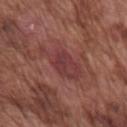| feature | finding |
|---|---|
| biopsy status | no biopsy performed (imaged during a skin exam) |
| image-analysis metrics | an average lesion color of about L≈39 a*≈26 b*≈21 (CIELAB), a lesion–skin lightness drop of about 7, and a normalized lesion–skin contrast near 6.5; border irregularity of about 5 on a 0–10 scale, internal color variation of about 3 on a 0–10 scale, and radial color variation of about 1 |
| acquisition | ~15 mm crop, total-body skin-cancer survey |
| lighting | white-light |
| body site | the right upper arm |
| lesion size | about 5.5 mm |
| patient | male, roughly 75 years of age |